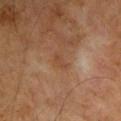Impression: No biopsy was performed on this lesion — it was imaged during a full skin examination and was not determined to be concerning. Context: The patient is a male in their 60s. Located on the right upper arm. This image is a 15 mm lesion crop taken from a total-body photograph.